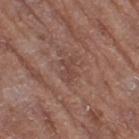No biopsy was performed on this lesion — it was imaged during a full skin examination and was not determined to be concerning. The subject is a female approximately 80 years of age. The total-body-photography lesion software estimated a mean CIELAB color near L≈44 a*≈19 b*≈24 and a normalized border contrast of about 5. The analysis additionally found a border-irregularity index near 4.5/10, a color-variation rating of about 2/10, and a peripheral color-asymmetry measure near 0.5. And it measured an automated nevus-likeness rating near 0 out of 100 and a detector confidence of about 80 out of 100 that the crop contains a lesion. A roughly 15 mm field-of-view crop from a total-body skin photograph. This is a white-light tile. The lesion is located on the right thigh.